The lesion's longest dimension is about 3.5 mm. The total-body-photography lesion software estimated a lesion area of about 9.5 mm². And it measured a border-irregularity rating of about 1/10, a color-variation rating of about 5/10, and a peripheral color-asymmetry measure near 1. A 15 mm close-up tile from a total-body photography series done for melanoma screening. A female patient, approximately 50 years of age. Located on the left forearm — 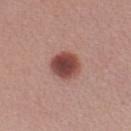The lesion was biopsied, and histopathology showed a dysplastic (Clark) nevus.